Cropped from a total-body skin-imaging series; the visible field is about 15 mm; on the lower back; a male subject aged approximately 55: 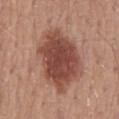An algorithmic analysis of the crop reported an area of roughly 32 mm², a shape eccentricity near 0.7, and two-axis asymmetry of about 0.15. It also reported a mean CIELAB color near L≈47 a*≈24 b*≈26, about 14 CIELAB-L* units darker than the surrounding skin, and a normalized lesion–skin contrast near 10. The analysis additionally found a color-variation rating of about 4.5/10 and radial color variation of about 1. The software also gave a classifier nevus-likeness of about 95/100 and a detector confidence of about 100 out of 100 that the crop contains a lesion.
Histopathologically confirmed as a dysplastic (Clark) nevus — a benign lesion.